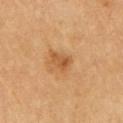{
  "biopsy_status": "not biopsied; imaged during a skin examination",
  "image": {
    "source": "total-body photography crop",
    "field_of_view_mm": 15
  },
  "lighting": "cross-polarized",
  "site": "right upper arm",
  "lesion_size": {
    "long_diameter_mm_approx": 2.5
  },
  "patient": {
    "sex": "male",
    "age_approx": 70
  }
}